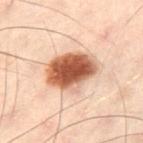notes = no biopsy performed (imaged during a skin exam); patient = male, roughly 50 years of age; image source = ~15 mm crop, total-body skin-cancer survey; body site = the left thigh; lighting = cross-polarized.